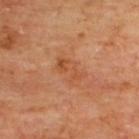Q: Is there a histopathology result?
A: no biopsy performed (imaged during a skin exam)
Q: Lesion location?
A: the upper back
Q: Patient demographics?
A: male, roughly 65 years of age
Q: What kind of image is this?
A: ~15 mm crop, total-body skin-cancer survey
Q: Lesion size?
A: about 3.5 mm
Q: Illumination type?
A: cross-polarized illumination